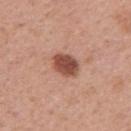Assessment: Imaged during a routine full-body skin examination; the lesion was not biopsied and no histopathology is available. Image and clinical context: Longest diameter approximately 3.5 mm. The total-body-photography lesion software estimated an eccentricity of roughly 0.7 and a symmetry-axis asymmetry near 0.15. A roughly 15 mm field-of-view crop from a total-body skin photograph. This is a white-light tile. Located on the right upper arm. The subject is a female aged 38 to 42.